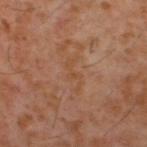Case summary:
• acquisition: ~15 mm crop, total-body skin-cancer survey
• automated metrics: a lesion area of about 4 mm², an outline eccentricity of about 0.9 (0 = round, 1 = elongated), and a symmetry-axis asymmetry near 0.7; a mean CIELAB color near L≈44 a*≈19 b*≈31, roughly 4 lightness units darker than nearby skin, and a normalized border contrast of about 4.5; an automated nevus-likeness rating near 0 out of 100
• subject: male, aged around 60
• diameter: ≈4 mm
• site: the back